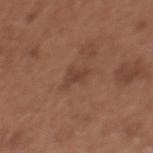biopsy_status: not biopsied; imaged during a skin examination
image:
  source: total-body photography crop
  field_of_view_mm: 15
patient:
  sex: female
  age_approx: 35
site: chest